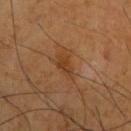This lesion was catalogued during total-body skin photography and was not selected for biopsy.
From the left upper arm.
The subject is a male aged approximately 70.
Longest diameter approximately 3 mm.
This is a cross-polarized tile.
A 15 mm close-up extracted from a 3D total-body photography capture.